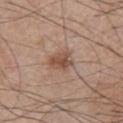Recorded during total-body skin imaging; not selected for excision or biopsy.
The lesion is located on the left arm.
A lesion tile, about 15 mm wide, cut from a 3D total-body photograph.
The lesion's longest dimension is about 3 mm.
A male subject aged 68–72.